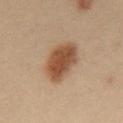No biopsy was performed on this lesion — it was imaged during a full skin examination and was not determined to be concerning. A 15 mm close-up tile from a total-body photography series done for melanoma screening. On the front of the torso. A female subject approximately 30 years of age.